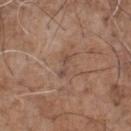The lesion was tiled from a total-body skin photograph and was not biopsied.
Measured at roughly 3 mm in maximum diameter.
Imaged with white-light lighting.
A 15 mm close-up tile from a total-body photography series done for melanoma screening.
From the front of the torso.
A male patient aged 68 to 72.